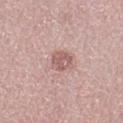The lesion was photographed on a routine skin check and not biopsied; there is no pathology result. Cropped from a total-body skin-imaging series; the visible field is about 15 mm. A female subject, in their mid- to late 60s. The lesion is located on the left thigh.